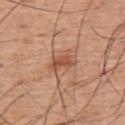<tbp_lesion>
<biopsy_status>not biopsied; imaged during a skin examination</biopsy_status>
<site>upper back</site>
<image>
  <source>total-body photography crop</source>
  <field_of_view_mm>15</field_of_view_mm>
</image>
<lighting>white-light</lighting>
<lesion_size>
  <long_diameter_mm_approx>3.5</long_diameter_mm_approx>
</lesion_size>
<patient>
  <sex>male</sex>
  <age_approx>55</age_approx>
</patient>
</tbp_lesion>